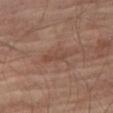follow-up = total-body-photography surveillance lesion; no biopsy | automated metrics = a border-irregularity index near 4.5/10 and a peripheral color-asymmetry measure near 0 | lesion diameter = ~4 mm (longest diameter) | image = ~15 mm tile from a whole-body skin photo | illumination = cross-polarized illumination | site = the right thigh | subject = male, about 70 years old.